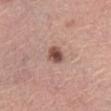The lesion was photographed on a routine skin check and not biopsied; there is no pathology result. The tile uses white-light illumination. The lesion's longest dimension is about 2.5 mm. Automated tile analysis of the lesion measured a border-irregularity rating of about 1.5/10, a within-lesion color-variation index near 3/10, and peripheral color asymmetry of about 1. It also reported a classifier nevus-likeness of about 95/100 and a detector confidence of about 100 out of 100 that the crop contains a lesion. A 15 mm close-up tile from a total-body photography series done for melanoma screening. A female patient, in their mid- to late 60s. On the abdomen.